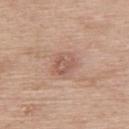Q: Was this lesion biopsied?
A: catalogued during a skin exam; not biopsied
Q: Patient demographics?
A: male, roughly 60 years of age
Q: What is the lesion's diameter?
A: ~3.5 mm (longest diameter)
Q: What is the anatomic site?
A: the upper back
Q: What did automated image analysis measure?
A: an average lesion color of about L≈57 a*≈21 b*≈26 (CIELAB) and roughly 8 lightness units darker than nearby skin; a nevus-likeness score of about 5/100 and a lesion-detection confidence of about 100/100
Q: How was this image acquired?
A: total-body-photography crop, ~15 mm field of view
Q: How was the tile lit?
A: white-light illumination